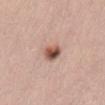Assessment: The lesion was photographed on a routine skin check and not biopsied; there is no pathology result. Background: Captured under white-light illumination. Longest diameter approximately 3 mm. A female subject aged approximately 45. Automated image analysis of the tile measured border irregularity of about 2 on a 0–10 scale. And it measured a nevus-likeness score of about 95/100 and a detector confidence of about 100 out of 100 that the crop contains a lesion. A 15 mm close-up extracted from a 3D total-body photography capture. On the lower back.